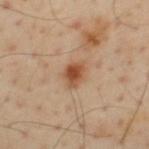Clinical impression: The lesion was tiled from a total-body skin photograph and was not biopsied. Clinical summary: The subject is a male about 55 years old. The lesion is located on the upper back. A close-up tile cropped from a whole-body skin photograph, about 15 mm across.